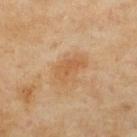anatomic site = the upper back | patient = female, aged 58–62 | imaging modality = total-body-photography crop, ~15 mm field of view | automated metrics = an average lesion color of about L≈52 a*≈19 b*≈35 (CIELAB) and about 7 CIELAB-L* units darker than the surrounding skin; a nevus-likeness score of about 20/100 and a lesion-detection confidence of about 100/100 | lesion diameter = ~3.5 mm (longest diameter).